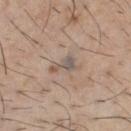Imaged during a routine full-body skin examination; the lesion was not biopsied and no histopathology is available. The lesion's longest dimension is about 3.5 mm. Automated image analysis of the tile measured an automated nevus-likeness rating near 0 out of 100 and lesion-presence confidence of about 75/100. A lesion tile, about 15 mm wide, cut from a 3D total-body photograph. A male patient, roughly 60 years of age. From the front of the torso.